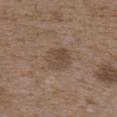| feature | finding |
|---|---|
| workup | no biopsy performed (imaged during a skin exam) |
| automated lesion analysis | a shape eccentricity near 0.75 and a symmetry-axis asymmetry near 0.15; an average lesion color of about L≈46 a*≈15 b*≈25 (CIELAB), about 8 CIELAB-L* units darker than the surrounding skin, and a normalized border contrast of about 6; a border-irregularity index near 2/10, a within-lesion color-variation index near 3.5/10, and radial color variation of about 1.5; a lesion-detection confidence of about 100/100 |
| site | the upper back |
| acquisition | ~15 mm tile from a whole-body skin photo |
| subject | female, aged approximately 35 |
| tile lighting | white-light |
| diameter | ~4 mm (longest diameter) |